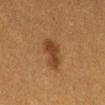A female patient, in their 40s. Located on the left thigh. Cropped from a whole-body photographic skin survey; the tile spans about 15 mm. Captured under cross-polarized illumination. Automated tile analysis of the lesion measured an eccentricity of roughly 0.8. And it measured internal color variation of about 2 on a 0–10 scale and a peripheral color-asymmetry measure near 0.5. And it measured a nevus-likeness score of about 100/100 and lesion-presence confidence of about 100/100.